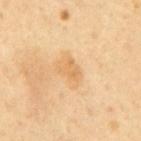{"biopsy_status": "not biopsied; imaged during a skin examination", "image": {"source": "total-body photography crop", "field_of_view_mm": 15}, "site": "mid back", "patient": {"sex": "male", "age_approx": 60}, "lesion_size": {"long_diameter_mm_approx": 2.5}, "lighting": "cross-polarized", "automated_metrics": {"border_irregularity_0_10": 3.5, "color_variation_0_10": 1.5, "peripheral_color_asymmetry": 0.5}}